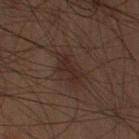Impression:
This lesion was catalogued during total-body skin photography and was not selected for biopsy.
Acquisition and patient details:
A close-up tile cropped from a whole-body skin photograph, about 15 mm across. From the left upper arm. The patient is a male in their 60s. Imaged with cross-polarized lighting.